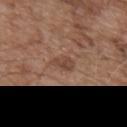Q: Was this lesion biopsied?
A: no biopsy performed (imaged during a skin exam)
Q: What is the anatomic site?
A: the upper back
Q: What are the patient's age and sex?
A: male, aged around 55
Q: What did automated image analysis measure?
A: a nevus-likeness score of about 0/100 and a detector confidence of about 100 out of 100 that the crop contains a lesion
Q: How was this image acquired?
A: 15 mm crop, total-body photography